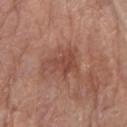Impression:
No biopsy was performed on this lesion — it was imaged during a full skin examination and was not determined to be concerning.
Image and clinical context:
The lesion-visualizer software estimated a border-irregularity rating of about 6.5/10, a within-lesion color-variation index near 3/10, and a peripheral color-asymmetry measure near 1. Located on the right forearm. Longest diameter approximately 5 mm. A female patient aged around 75. Cropped from a total-body skin-imaging series; the visible field is about 15 mm.